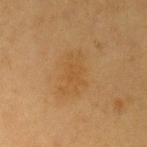Case summary:
– notes: no biopsy performed (imaged during a skin exam)